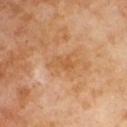Captured during whole-body skin photography for melanoma surveillance; the lesion was not biopsied.
Measured at roughly 3.5 mm in maximum diameter.
An algorithmic analysis of the crop reported a lesion area of about 5 mm², an outline eccentricity of about 0.85 (0 = round, 1 = elongated), and a shape-asymmetry score of about 0.3 (0 = symmetric). The software also gave a color-variation rating of about 3/10 and a peripheral color-asymmetry measure near 1.5. And it measured a nevus-likeness score of about 0/100 and lesion-presence confidence of about 100/100.
A male subject, approximately 65 years of age.
This is a cross-polarized tile.
A region of skin cropped from a whole-body photographic capture, roughly 15 mm wide.